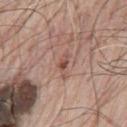{"biopsy_status": "not biopsied; imaged during a skin examination", "site": "chest", "automated_metrics": {"cielab_L": 50, "cielab_a": 22, "cielab_b": 26, "vs_skin_darker_L": 10.0, "vs_skin_contrast_norm": 7.5, "border_irregularity_0_10": 5.5, "peripheral_color_asymmetry": 0.0, "nevus_likeness_0_100": 10, "lesion_detection_confidence_0_100": 100}, "lighting": "white-light", "image": {"source": "total-body photography crop", "field_of_view_mm": 15}, "lesion_size": {"long_diameter_mm_approx": 3.0}, "patient": {"sex": "male", "age_approx": 80}}